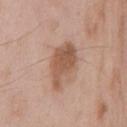Impression: The lesion was tiled from a total-body skin photograph and was not biopsied. Background: From the front of the torso. A male patient, aged approximately 65. A 15 mm crop from a total-body photograph taken for skin-cancer surveillance. About 5.5 mm across. Captured under white-light illumination.